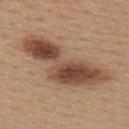Imaged during a routine full-body skin examination; the lesion was not biopsied and no histopathology is available. From the upper back. A 15 mm close-up extracted from a 3D total-body photography capture. A female patient approximately 30 years of age.